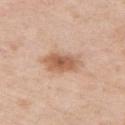Acquisition and patient details:
The lesion-visualizer software estimated a lesion area of about 9.5 mm², a shape eccentricity near 0.75, and a shape-asymmetry score of about 0.15 (0 = symmetric). And it measured about 12 CIELAB-L* units darker than the surrounding skin and a normalized border contrast of about 8. It also reported an automated nevus-likeness rating near 85 out of 100. A female subject aged 38–42. The lesion is on the right upper arm. Cropped from a whole-body photographic skin survey; the tile spans about 15 mm. The tile uses white-light illumination. The recorded lesion diameter is about 4.5 mm.